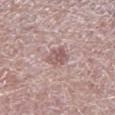The lesion is located on the left lower leg.
A male subject, in their mid- to late 60s.
This is a white-light tile.
Cropped from a whole-body photographic skin survey; the tile spans about 15 mm.
About 3 mm across.